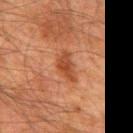{
  "biopsy_status": "not biopsied; imaged during a skin examination",
  "site": "back",
  "image": {
    "source": "total-body photography crop",
    "field_of_view_mm": 15
  },
  "patient": {
    "sex": "male",
    "age_approx": 60
  }
}